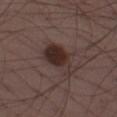The patient is a male in their 40s.
On the left thigh.
Cropped from a total-body skin-imaging series; the visible field is about 15 mm.